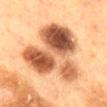Findings:
* notes — total-body-photography surveillance lesion; no biopsy
* acquisition — total-body-photography crop, ~15 mm field of view
* lesion size — about 8.5 mm
* anatomic site — the mid back
* image-analysis metrics — a footprint of about 43 mm² and a shape-asymmetry score of about 0.5 (0 = symmetric); a lesion color around L≈47 a*≈21 b*≈32 in CIELAB, a lesion–skin lightness drop of about 17, and a normalized lesion–skin contrast near 12; an automated nevus-likeness rating near 0 out of 100
* subject — female, in their mid-50s
* lighting — cross-polarized illumination